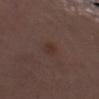{"biopsy_status": "not biopsied; imaged during a skin examination", "image": {"source": "total-body photography crop", "field_of_view_mm": 15}, "lighting": "white-light", "patient": {"sex": "male", "age_approx": 70}, "automated_metrics": {"eccentricity": 0.8, "shape_asymmetry": 0.3, "border_irregularity_0_10": 2.5, "color_variation_0_10": 1.5, "peripheral_color_asymmetry": 0.5}, "site": "left forearm", "lesion_size": {"long_diameter_mm_approx": 2.5}}